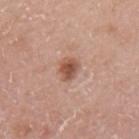  biopsy_status: not biopsied; imaged during a skin examination
  lighting: white-light
  patient:
    sex: female
    age_approx: 30
  site: upper back
  lesion_size:
    long_diameter_mm_approx: 3.0
  image:
    source: total-body photography crop
    field_of_view_mm: 15